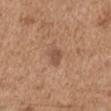notes: no biopsy performed (imaged during a skin exam)
acquisition: total-body-photography crop, ~15 mm field of view
body site: the arm
tile lighting: white-light illumination
size: about 2.5 mm
automated metrics: a shape eccentricity near 0.65 and a symmetry-axis asymmetry near 0.3; a mean CIELAB color near L≈51 a*≈20 b*≈29, roughly 8 lightness units darker than nearby skin, and a lesion-to-skin contrast of about 6 (normalized; higher = more distinct); a border-irregularity rating of about 2.5/10 and a color-variation rating of about 1/10
patient: male, approximately 65 years of age